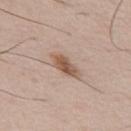Captured during whole-body skin photography for melanoma surveillance; the lesion was not biopsied. A 15 mm close-up extracted from a 3D total-body photography capture. The subject is a male aged 53–57. The total-body-photography lesion software estimated a footprint of about 6.5 mm², a shape eccentricity near 0.9, and a symmetry-axis asymmetry near 0.25. And it measured an average lesion color of about L≈56 a*≈17 b*≈28 (CIELAB) and roughly 12 lightness units darker than nearby skin. From the chest. About 4.5 mm across.